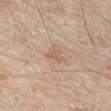The lesion was photographed on a routine skin check and not biopsied; there is no pathology result. Imaged with white-light lighting. The subject is a male aged around 80. Located on the abdomen. A 15 mm crop from a total-body photograph taken for skin-cancer surveillance.